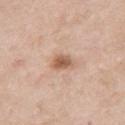workup = imaged on a skin check; not biopsied | site = the left upper arm | lesion size = about 2.5 mm | tile lighting = white-light | patient = male, aged around 50 | image = ~15 mm tile from a whole-body skin photo.